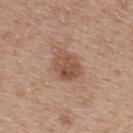Clinical impression: Imaged during a routine full-body skin examination; the lesion was not biopsied and no histopathology is available. Background: Automated image analysis of the tile measured an area of roughly 9.5 mm², an eccentricity of roughly 0.6, and a shape-asymmetry score of about 0.2 (0 = symmetric). The software also gave an average lesion color of about L≈51 a*≈20 b*≈29 (CIELAB) and a lesion–skin lightness drop of about 10. The lesion is located on the back. This image is a 15 mm lesion crop taken from a total-body photograph. This is a white-light tile. About 4 mm across. A female patient, aged around 40.